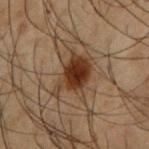{"biopsy_status": "not biopsied; imaged during a skin examination", "site": "left upper arm", "patient": {"sex": "male", "age_approx": 50}, "lighting": "cross-polarized", "lesion_size": {"long_diameter_mm_approx": 4.5}, "image": {"source": "total-body photography crop", "field_of_view_mm": 15}}